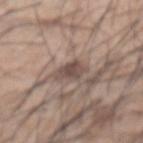Imaged with white-light lighting. From the front of the torso. This image is a 15 mm lesion crop taken from a total-body photograph. Longest diameter approximately 3 mm. A male patient, in their mid- to late 50s.